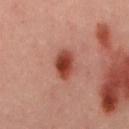<tbp_lesion>
  <biopsy_status>not biopsied; imaged during a skin examination</biopsy_status>
  <site>mid back</site>
  <lesion_size>
    <long_diameter_mm_approx>4.0</long_diameter_mm_approx>
  </lesion_size>
  <image>
    <source>total-body photography crop</source>
    <field_of_view_mm>15</field_of_view_mm>
  </image>
  <patient>
    <sex>male</sex>
    <age_approx>40</age_approx>
  </patient>
</tbp_lesion>